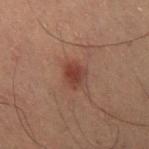Assessment: No biopsy was performed on this lesion — it was imaged during a full skin examination and was not determined to be concerning. Context: Approximately 3 mm at its widest. The tile uses cross-polarized illumination. The lesion is located on the leg. A roughly 15 mm field-of-view crop from a total-body skin photograph. The subject is a male approximately 60 years of age.